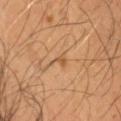Part of a total-body skin-imaging series; this lesion was reviewed on a skin check and was not flagged for biopsy. A lesion tile, about 15 mm wide, cut from a 3D total-body photograph. On the head or neck. A male patient aged 53–57. Imaged with cross-polarized lighting. Longest diameter approximately 2.5 mm.